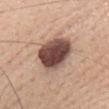follow-up: no biopsy performed (imaged during a skin exam) | subject: female, aged 43 to 47 | tile lighting: white-light | location: the head or neck | image: total-body-photography crop, ~15 mm field of view | TBP lesion metrics: a lesion area of about 18 mm², an outline eccentricity of about 0.55 (0 = round, 1 = elongated), and a symmetry-axis asymmetry near 0.2; a border-irregularity index near 2/10, internal color variation of about 7 on a 0–10 scale, and radial color variation of about 2 | diameter: ~5 mm (longest diameter).